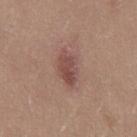biopsy status: no biopsy performed (imaged during a skin exam)
diameter: ~4.5 mm (longest diameter)
anatomic site: the front of the torso
imaging modality: ~15 mm tile from a whole-body skin photo
tile lighting: white-light illumination
patient: male, in their mid- to late 20s